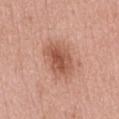The lesion was tiled from a total-body skin photograph and was not biopsied. The patient is a female aged 68 to 72. Automated tile analysis of the lesion measured an automated nevus-likeness rating near 90 out of 100 and a lesion-detection confidence of about 100/100. The lesion's longest dimension is about 5 mm. Imaged with white-light lighting. This image is a 15 mm lesion crop taken from a total-body photograph. Located on the front of the torso.